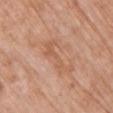<case>
<biopsy_status>not biopsied; imaged during a skin examination</biopsy_status>
<site>chest</site>
<automated_metrics>
  <vs_skin_darker_L>6.0</vs_skin_darker_L>
  <vs_skin_contrast_norm>5.0</vs_skin_contrast_norm>
  <border_irregularity_0_10>8.0</border_irregularity_0_10>
  <color_variation_0_10>1.0</color_variation_0_10>
  <nevus_likeness_0_100>0</nevus_likeness_0_100>
  <lesion_detection_confidence_0_100>100</lesion_detection_confidence_0_100>
</automated_metrics>
<image>
  <source>total-body photography crop</source>
  <field_of_view_mm>15</field_of_view_mm>
</image>
<lesion_size>
  <long_diameter_mm_approx>5.5</long_diameter_mm_approx>
</lesion_size>
<lighting>white-light</lighting>
<patient>
  <sex>female</sex>
  <age_approx>60</age_approx>
</patient>
</case>